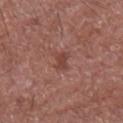biopsy_status: not biopsied; imaged during a skin examination
image:
  source: total-body photography crop
  field_of_view_mm: 15
patient:
  sex: male
  age_approx: 70
site: right upper arm
lesion_size:
  long_diameter_mm_approx: 2.5